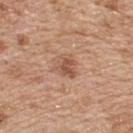This lesion was catalogued during total-body skin photography and was not selected for biopsy. A male subject aged around 70. This image is a 15 mm lesion crop taken from a total-body photograph. The lesion is located on the upper back.